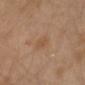Image and clinical context:
A male patient approximately 30 years of age. On the left arm. A 15 mm crop from a total-body photograph taken for skin-cancer surveillance.